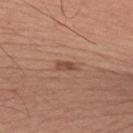This lesion was catalogued during total-body skin photography and was not selected for biopsy.
This image is a 15 mm lesion crop taken from a total-body photograph.
From the upper back.
A male patient aged 33–37.